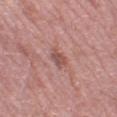Q: What kind of image is this?
A: ~15 mm tile from a whole-body skin photo
Q: Illumination type?
A: white-light illumination
Q: How large is the lesion?
A: ≈2.5 mm
Q: What is the anatomic site?
A: the left thigh
Q: Who is the patient?
A: female, roughly 40 years of age